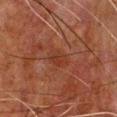Clinical impression: The lesion was tiled from a total-body skin photograph and was not biopsied. Context: A region of skin cropped from a whole-body photographic capture, roughly 15 mm wide. The tile uses cross-polarized illumination. A male patient in their mid-60s. From the chest.